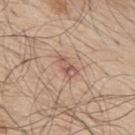  biopsy_status: not biopsied; imaged during a skin examination
  site: upper back
  patient:
    sex: male
    age_approx: 70
  lesion_size:
    long_diameter_mm_approx: 3.0
  lighting: white-light
  image:
    source: total-body photography crop
    field_of_view_mm: 15
  automated_metrics:
    area_mm2_approx: 3.5
    eccentricity: 0.85
    shape_asymmetry: 0.4
    color_variation_0_10: 3.0
    peripheral_color_asymmetry: 1.0